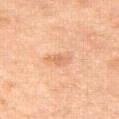The lesion was photographed on a routine skin check and not biopsied; there is no pathology result. The tile uses cross-polarized illumination. On the right thigh. A close-up tile cropped from a whole-body skin photograph, about 15 mm across. The patient is a female about 55 years old. An algorithmic analysis of the crop reported a footprint of about 3 mm² and an eccentricity of roughly 0.9. The analysis additionally found a mean CIELAB color near L≈58 a*≈21 b*≈33, about 8 CIELAB-L* units darker than the surrounding skin, and a lesion-to-skin contrast of about 5.5 (normalized; higher = more distinct). Measured at roughly 3 mm in maximum diameter.